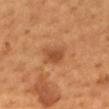  biopsy_status: not biopsied; imaged during a skin examination
  site: mid back
  image:
    source: total-body photography crop
    field_of_view_mm: 15
  patient:
    sex: male
    age_approx: 55